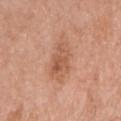| feature | finding |
|---|---|
| diameter | about 3.5 mm |
| subject | female, about 60 years old |
| lighting | white-light illumination |
| site | the right upper arm |
| imaging modality | ~15 mm tile from a whole-body skin photo |